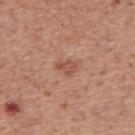No biopsy was performed on this lesion — it was imaged during a full skin examination and was not determined to be concerning. A roughly 15 mm field-of-view crop from a total-body skin photograph. This is a white-light tile. The lesion is on the upper back. The lesion-visualizer software estimated a footprint of about 4 mm² and a shape-asymmetry score of about 0.4 (0 = symmetric). It also reported border irregularity of about 3.5 on a 0–10 scale, internal color variation of about 1 on a 0–10 scale, and a peripheral color-asymmetry measure near 0.5. The analysis additionally found a nevus-likeness score of about 5/100 and a detector confidence of about 100 out of 100 that the crop contains a lesion. A male patient, aged 68–72.